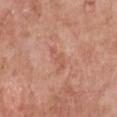<lesion>
  <biopsy_status>not biopsied; imaged during a skin examination</biopsy_status>
  <patient>
    <sex>female</sex>
    <age_approx>60</age_approx>
  </patient>
  <site>chest</site>
  <image>
    <source>total-body photography crop</source>
    <field_of_view_mm>15</field_of_view_mm>
  </image>
</lesion>